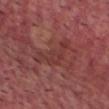Findings:
• workup — imaged on a skin check; not biopsied
• patient — male, in their mid-70s
• image-analysis metrics — border irregularity of about 9 on a 0–10 scale and radial color variation of about 0.5; a classifier nevus-likeness of about 0/100 and a detector confidence of about 75 out of 100 that the crop contains a lesion
• image source — total-body-photography crop, ~15 mm field of view
• site — the front of the torso
• size — ~5 mm (longest diameter)
• lighting — white-light